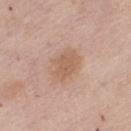Cropped from a total-body skin-imaging series; the visible field is about 15 mm. Located on the left thigh. A female patient about 65 years old.